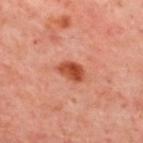Recorded during total-body skin imaging; not selected for excision or biopsy.
The lesion is on the upper back.
A subject about 55 years old.
Captured under cross-polarized illumination.
About 3.5 mm across.
A 15 mm close-up extracted from a 3D total-body photography capture.
Automated image analysis of the tile measured an area of roughly 6 mm², an outline eccentricity of about 0.8 (0 = round, 1 = elongated), and a shape-asymmetry score of about 0.25 (0 = symmetric). The software also gave an average lesion color of about L≈50 a*≈32 b*≈36 (CIELAB), roughly 13 lightness units darker than nearby skin, and a normalized border contrast of about 9.5. The analysis additionally found an automated nevus-likeness rating near 95 out of 100 and lesion-presence confidence of about 100/100.